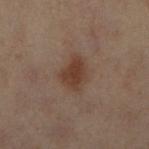Q: Is there a histopathology result?
A: no biopsy performed (imaged during a skin exam)
Q: What lighting was used for the tile?
A: cross-polarized
Q: Lesion location?
A: the left leg
Q: How was this image acquired?
A: total-body-photography crop, ~15 mm field of view
Q: Lesion size?
A: ~3.5 mm (longest diameter)
Q: What are the patient's age and sex?
A: female, about 55 years old